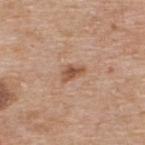Findings:
– notes · no biopsy performed (imaged during a skin exam)
– tile lighting · white-light illumination
– location · the upper back
– image source · 15 mm crop, total-body photography
– patient · male, about 60 years old